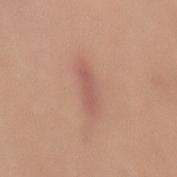Assessment: Part of a total-body skin-imaging series; this lesion was reviewed on a skin check and was not flagged for biopsy. Image and clinical context: A female subject roughly 55 years of age. Measured at roughly 4.5 mm in maximum diameter. Cropped from a total-body skin-imaging series; the visible field is about 15 mm. On the right thigh.